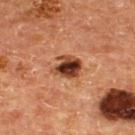The lesion was photographed on a routine skin check and not biopsied; there is no pathology result. A region of skin cropped from a whole-body photographic capture, roughly 15 mm wide. The tile uses cross-polarized illumination. Measured at roughly 3.5 mm in maximum diameter. The subject is a male aged around 65. From the upper back.